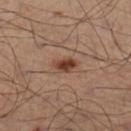biopsy status: no biopsy performed (imaged during a skin exam) | illumination: cross-polarized illumination | image: ~15 mm crop, total-body skin-cancer survey | subject: male, in their 50s | body site: the left leg | automated lesion analysis: a lesion area of about 4.5 mm², a shape eccentricity near 0.8, and a shape-asymmetry score of about 0.2 (0 = symmetric); an average lesion color of about L≈41 a*≈20 b*≈29 (CIELAB) and about 12 CIELAB-L* units darker than the surrounding skin; a detector confidence of about 100 out of 100 that the crop contains a lesion.